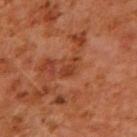biopsy_status: not biopsied; imaged during a skin examination
image:
  source: total-body photography crop
  field_of_view_mm: 15
patient:
  sex: male
  age_approx: 60
lesion_size:
  long_diameter_mm_approx: 2.5
automated_metrics:
  area_mm2_approx: 3.0
  eccentricity: 0.8
  shape_asymmetry: 0.55
  border_irregularity_0_10: 5.0
  peripheral_color_asymmetry: 0.5
  nevus_likeness_0_100: 0
  lesion_detection_confidence_0_100: 100
lighting: cross-polarized
site: right upper arm